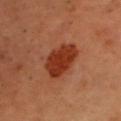Q: Is there a histopathology result?
A: imaged on a skin check; not biopsied
Q: Lesion location?
A: the head or neck
Q: Illumination type?
A: cross-polarized illumination
Q: Lesion size?
A: ~5.5 mm (longest diameter)
Q: Patient demographics?
A: male, in their mid- to late 50s
Q: How was this image acquired?
A: ~15 mm crop, total-body skin-cancer survey